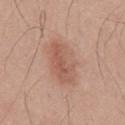biopsy_status: not biopsied; imaged during a skin examination
patient:
  sex: male
  age_approx: 30
site: chest
image:
  source: total-body photography crop
  field_of_view_mm: 15